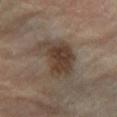notes: no biopsy performed (imaged during a skin exam)
image-analysis metrics: a lesion color around L≈37 a*≈13 b*≈22 in CIELAB, about 10 CIELAB-L* units darker than the surrounding skin, and a lesion-to-skin contrast of about 9 (normalized; higher = more distinct); a border-irregularity index near 4/10, a within-lesion color-variation index near 4.5/10, and peripheral color asymmetry of about 1.5; a nevus-likeness score of about 80/100 and a detector confidence of about 100 out of 100 that the crop contains a lesion
site: the right leg
lesion diameter: ~5.5 mm (longest diameter)
image: ~15 mm crop, total-body skin-cancer survey
subject: female, in their mid-60s
tile lighting: cross-polarized illumination